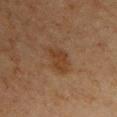<lesion>
  <biopsy_status>not biopsied; imaged during a skin examination</biopsy_status>
  <lesion_size>
    <long_diameter_mm_approx>4.0</long_diameter_mm_approx>
  </lesion_size>
  <automated_metrics>
    <area_mm2_approx>7.5</area_mm2_approx>
    <vs_skin_darker_L>6.0</vs_skin_darker_L>
    <vs_skin_contrast_norm>6.5</vs_skin_contrast_norm>
    <nevus_likeness_0_100>10</nevus_likeness_0_100>
    <lesion_detection_confidence_0_100>100</lesion_detection_confidence_0_100>
  </automated_metrics>
  <image>
    <source>total-body photography crop</source>
    <field_of_view_mm>15</field_of_view_mm>
  </image>
  <lighting>cross-polarized</lighting>
  <site>chest</site>
  <patient>
    <sex>male</sex>
    <age_approx>65</age_approx>
  </patient>
</lesion>